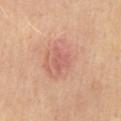The lesion was photographed on a routine skin check and not biopsied; there is no pathology result.
This image is a 15 mm lesion crop taken from a total-body photograph.
From the abdomen.
The lesion's longest dimension is about 3.5 mm.
A female patient, roughly 50 years of age.
The tile uses cross-polarized illumination.